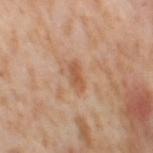patient: female, in their mid- to late 50s; image source: ~15 mm crop, total-body skin-cancer survey; anatomic site: the right thigh; tile lighting: cross-polarized illumination.